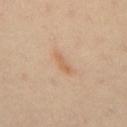Imaged during a routine full-body skin examination; the lesion was not biopsied and no histopathology is available.
Imaged with cross-polarized lighting.
Approximately 3 mm at its widest.
The patient is a male aged 43 to 47.
A 15 mm close-up tile from a total-body photography series done for melanoma screening.
Located on the mid back.